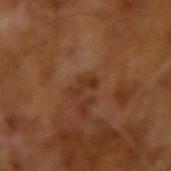notes: catalogued during a skin exam; not biopsied
diameter: about 2.5 mm
illumination: cross-polarized
subject: male, aged 63–67
image source: ~15 mm crop, total-body skin-cancer survey